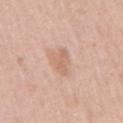<record>
<lighting>white-light</lighting>
<automated_metrics>
  <border_irregularity_0_10>4.0</border_irregularity_0_10>
  <color_variation_0_10>1.5</color_variation_0_10>
  <lesion_detection_confidence_0_100>100</lesion_detection_confidence_0_100>
</automated_metrics>
<site>arm</site>
<lesion_size>
  <long_diameter_mm_approx>3.5</long_diameter_mm_approx>
</lesion_size>
<image>
  <source>total-body photography crop</source>
  <field_of_view_mm>15</field_of_view_mm>
</image>
<patient>
  <sex>male</sex>
  <age_approx>55</age_approx>
</patient>
</record>